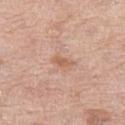workup: total-body-photography surveillance lesion; no biopsy | patient: female, roughly 65 years of age | location: the left thigh | acquisition: 15 mm crop, total-body photography.